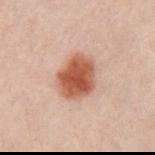Located on the chest. Captured under cross-polarized illumination. A 15 mm close-up extracted from a 3D total-body photography capture. A male subject about 50 years old.